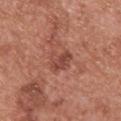<lesion>
<biopsy_status>not biopsied; imaged during a skin examination</biopsy_status>
<site>upper back</site>
<image>
  <source>total-body photography crop</source>
  <field_of_view_mm>15</field_of_view_mm>
</image>
<patient>
  <sex>female</sex>
  <age_approx>40</age_approx>
</patient>
</lesion>